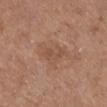Q: What is the imaging modality?
A: ~15 mm tile from a whole-body skin photo
Q: What is the anatomic site?
A: the right lower leg
Q: Illumination type?
A: white-light
Q: Automated lesion metrics?
A: an outline eccentricity of about 0.9 (0 = round, 1 = elongated); a border-irregularity index near 5.5/10, a within-lesion color-variation index near 0.5/10, and a peripheral color-asymmetry measure near 0
Q: Lesion size?
A: ≈3.5 mm
Q: What are the patient's age and sex?
A: female, aged 38 to 42